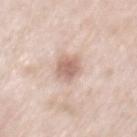biopsy status=no biopsy performed (imaged during a skin exam).